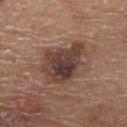| field | value |
|---|---|
| workup | total-body-photography surveillance lesion; no biopsy |
| image-analysis metrics | an average lesion color of about L≈40 a*≈18 b*≈23 (CIELAB), roughly 12 lightness units darker than nearby skin, and a normalized lesion–skin contrast near 10 |
| imaging modality | ~15 mm crop, total-body skin-cancer survey |
| lesion diameter | ~6 mm (longest diameter) |
| tile lighting | white-light illumination |
| subject | male, about 80 years old |
| site | the front of the torso |